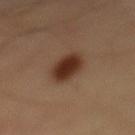| field | value |
|---|---|
| workup | no biopsy performed (imaged during a skin exam) |
| image-analysis metrics | an outline eccentricity of about 0.8 (0 = round, 1 = elongated) and two-axis asymmetry of about 0.15; a normalized lesion–skin contrast near 11.5; a border-irregularity rating of about 2/10, a within-lesion color-variation index near 3.5/10, and peripheral color asymmetry of about 1 |
| imaging modality | total-body-photography crop, ~15 mm field of view |
| patient | male, aged approximately 55 |
| lesion size | ~4.5 mm (longest diameter) |
| anatomic site | the mid back |
| illumination | cross-polarized |